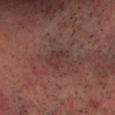Findings:
- workup — catalogued during a skin exam; not biopsied
- patient — aged 53–57
- TBP lesion metrics — a lesion area of about 4.5 mm² and a symmetry-axis asymmetry near 0.35
- imaging modality — total-body-photography crop, ~15 mm field of view
- illumination — cross-polarized
- site — the head or neck